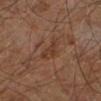biopsy status=catalogued during a skin exam; not biopsied
patient=male, roughly 60 years of age
anatomic site=the right leg
imaging modality=15 mm crop, total-body photography